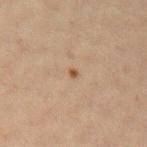Case summary:
* notes — catalogued during a skin exam; not biopsied
* location — the left upper arm
* image — total-body-photography crop, ~15 mm field of view
* patient — female, about 40 years old